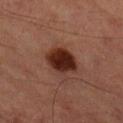Case summary:
* follow-up · total-body-photography surveillance lesion; no biopsy
* body site · the right lower leg
* subject · male, aged 83 or over
* image · ~15 mm crop, total-body skin-cancer survey